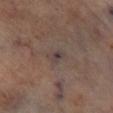Impression:
Captured during whole-body skin photography for melanoma surveillance; the lesion was not biopsied.
Image and clinical context:
The recorded lesion diameter is about 2.5 mm. Captured under cross-polarized illumination. Automated image analysis of the tile measured an eccentricity of roughly 0.85 and two-axis asymmetry of about 0.3. And it measured a border-irregularity index near 2.5/10 and peripheral color asymmetry of about 2. The lesion is located on the right lower leg. A region of skin cropped from a whole-body photographic capture, roughly 15 mm wide.